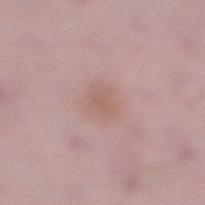  biopsy_status: not biopsied; imaged during a skin examination
  image:
    source: total-body photography crop
    field_of_view_mm: 15
  site: right lower leg
  patient:
    sex: male
    age_approx: 50
  lesion_size:
    long_diameter_mm_approx: 3.0
  automated_metrics:
    area_mm2_approx: 6.0
    nevus_likeness_0_100: 5